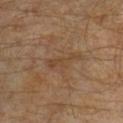body site: the left leg
acquisition: 15 mm crop, total-body photography
patient: male, in their 30s